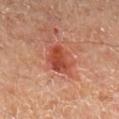Context:
The tile uses cross-polarized illumination. Measured at roughly 3.5 mm in maximum diameter. A male subject roughly 55 years of age. On the right lower leg. Cropped from a whole-body photographic skin survey; the tile spans about 15 mm.